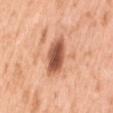Findings:
* illumination — white-light illumination
* image source — ~15 mm crop, total-body skin-cancer survey
* location — the arm
* automated lesion analysis — a lesion area of about 9.5 mm², a shape eccentricity near 0.7, and a symmetry-axis asymmetry near 0.2; a mean CIELAB color near L≈55 a*≈26 b*≈34 and a lesion–skin lightness drop of about 17; radial color variation of about 1.5; a detector confidence of about 100 out of 100 that the crop contains a lesion
* diameter — ~4 mm (longest diameter)
* patient — male, roughly 45 years of age